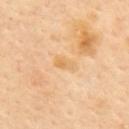{
  "biopsy_status": "not biopsied; imaged during a skin examination",
  "automated_metrics": {
    "cielab_L": 73,
    "cielab_a": 19,
    "cielab_b": 45,
    "vs_skin_darker_L": 7.0,
    "vs_skin_contrast_norm": 5.5,
    "nevus_likeness_0_100": 0,
    "lesion_detection_confidence_0_100": 100
  },
  "patient": {
    "sex": "female",
    "age_approx": 60
  },
  "image": {
    "source": "total-body photography crop",
    "field_of_view_mm": 15
  },
  "site": "upper back"
}